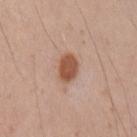No biopsy was performed on this lesion — it was imaged during a full skin examination and was not determined to be concerning. A male patient, in their mid- to late 30s. Measured at roughly 3.5 mm in maximum diameter. A region of skin cropped from a whole-body photographic capture, roughly 15 mm wide. This is a white-light tile. The lesion is located on the left upper arm.